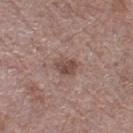Clinical impression: The lesion was photographed on a routine skin check and not biopsied; there is no pathology result. Acquisition and patient details: About 2.5 mm across. Captured under white-light illumination. A male subject approximately 70 years of age. Cropped from a total-body skin-imaging series; the visible field is about 15 mm. The lesion is on the left thigh.